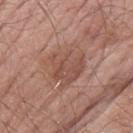Notes:
* follow-up — no biopsy performed (imaged during a skin exam)
* tile lighting — white-light illumination
* body site — the left forearm
* subject — male, aged 58–62
* image source — ~15 mm tile from a whole-body skin photo